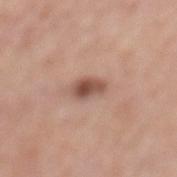Impression: Imaged during a routine full-body skin examination; the lesion was not biopsied and no histopathology is available. Context: This is a white-light tile. A region of skin cropped from a whole-body photographic capture, roughly 15 mm wide. An algorithmic analysis of the crop reported a lesion area of about 5.5 mm², a shape eccentricity near 0.8, and a shape-asymmetry score of about 0.25 (0 = symmetric). And it measured a lesion–skin lightness drop of about 13 and a normalized border contrast of about 9. And it measured an automated nevus-likeness rating near 90 out of 100 and a lesion-detection confidence of about 100/100. From the leg. The recorded lesion diameter is about 3.5 mm. A female patient aged around 70.